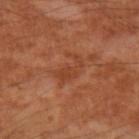The lesion was tiled from a total-body skin photograph and was not biopsied.
Located on the left upper arm.
A male patient, aged 53 to 57.
Longest diameter approximately 4 mm.
Cropped from a total-body skin-imaging series; the visible field is about 15 mm.